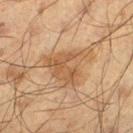follow-up = no biopsy performed (imaged during a skin exam) | imaging modality = 15 mm crop, total-body photography | subject = male, in their 60s | lesion diameter = ~7 mm (longest diameter) | illumination = cross-polarized | anatomic site = the left thigh.